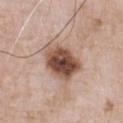Context:
A male subject, roughly 50 years of age. Automated image analysis of the tile measured a footprint of about 16 mm², an eccentricity of roughly 0.65, and a shape-asymmetry score of about 0.2 (0 = symmetric). The analysis additionally found an average lesion color of about L≈50 a*≈19 b*≈27 (CIELAB), roughly 18 lightness units darker than nearby skin, and a lesion-to-skin contrast of about 11.5 (normalized; higher = more distinct). And it measured a border-irregularity index near 2/10, a within-lesion color-variation index near 9/10, and a peripheral color-asymmetry measure near 3. The software also gave an automated nevus-likeness rating near 75 out of 100 and lesion-presence confidence of about 100/100. On the chest. Cropped from a whole-body photographic skin survey; the tile spans about 15 mm.